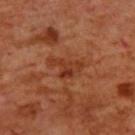follow-up = catalogued during a skin exam; not biopsied